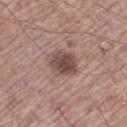| field | value |
|---|---|
| workup | imaged on a skin check; not biopsied |
| subject | male, aged 68–72 |
| site | the left thigh |
| image source | 15 mm crop, total-body photography |
| lighting | white-light illumination |